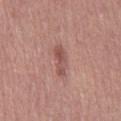Findings:
• biopsy status: no biopsy performed (imaged during a skin exam)
• lighting: white-light illumination
• image: total-body-photography crop, ~15 mm field of view
• patient: female, aged 58 to 62
• automated metrics: an area of roughly 5 mm², an outline eccentricity of about 0.95 (0 = round, 1 = elongated), and two-axis asymmetry of about 0.3; a border-irregularity index near 4/10 and peripheral color asymmetry of about 0; a classifier nevus-likeness of about 10/100
• lesion diameter: ~4 mm (longest diameter)
• anatomic site: the leg